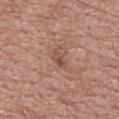Assessment: No biopsy was performed on this lesion — it was imaged during a full skin examination and was not determined to be concerning. Acquisition and patient details: Automated tile analysis of the lesion measured a detector confidence of about 100 out of 100 that the crop contains a lesion. On the upper back. About 2.5 mm across. Cropped from a whole-body photographic skin survey; the tile spans about 15 mm. A male subject, approximately 70 years of age.